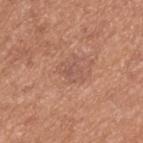Recorded during total-body skin imaging; not selected for excision or biopsy.
A male patient in their mid-50s.
Cropped from a whole-body photographic skin survey; the tile spans about 15 mm.
From the upper back.
The tile uses white-light illumination.